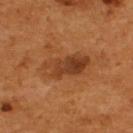biopsy status=catalogued during a skin exam; not biopsied
subject=male, aged approximately 55
image=~15 mm crop, total-body skin-cancer survey
diameter=~5.5 mm (longest diameter)
lighting=cross-polarized
site=the upper back
TBP lesion metrics=a footprint of about 12 mm², an eccentricity of roughly 0.9, and two-axis asymmetry of about 0.15; a color-variation rating of about 6.5/10 and peripheral color asymmetry of about 2.5